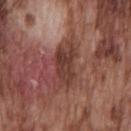{"biopsy_status": "not biopsied; imaged during a skin examination", "site": "chest", "patient": {"sex": "male", "age_approx": 75}, "lighting": "white-light", "image": {"source": "total-body photography crop", "field_of_view_mm": 15}, "automated_metrics": {"area_mm2_approx": 12.0, "eccentricity": 0.9, "shape_asymmetry": 0.3, "cielab_L": 39, "cielab_a": 22, "cielab_b": 24, "vs_skin_darker_L": 10.0, "vs_skin_contrast_norm": 8.0, "color_variation_0_10": 4.5, "peripheral_color_asymmetry": 1.5, "lesion_detection_confidence_0_100": 65}, "lesion_size": {"long_diameter_mm_approx": 5.5}}